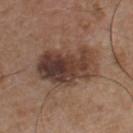<lesion>
<biopsy_status>not biopsied; imaged during a skin examination</biopsy_status>
<site>chest</site>
<patient>
  <sex>male</sex>
  <age_approx>55</age_approx>
</patient>
<lesion_size>
  <long_diameter_mm_approx>7.0</long_diameter_mm_approx>
</lesion_size>
<image>
  <source>total-body photography crop</source>
  <field_of_view_mm>15</field_of_view_mm>
</image>
<lighting>white-light</lighting>
</lesion>